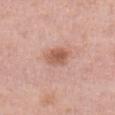patient:
  sex: female
  age_approx: 40
image:
  source: total-body photography crop
  field_of_view_mm: 15
site: abdomen
lighting: white-light
lesion_size:
  long_diameter_mm_approx: 3.5